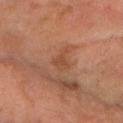{"biopsy_status": "not biopsied; imaged during a skin examination", "automated_metrics": {"area_mm2_approx": 4.0, "eccentricity": 0.8, "shape_asymmetry": 0.45, "vs_skin_contrast_norm": 5.0, "border_irregularity_0_10": 5.0, "color_variation_0_10": 1.0, "peripheral_color_asymmetry": 0.5, "nevus_likeness_0_100": 0, "lesion_detection_confidence_0_100": 100}, "image": {"source": "total-body photography crop", "field_of_view_mm": 15}, "lighting": "cross-polarized", "site": "arm", "patient": {"sex": "female", "age_approx": 70}, "lesion_size": {"long_diameter_mm_approx": 3.0}}